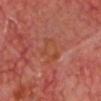Q: How was the tile lit?
A: cross-polarized illumination
Q: What are the patient's age and sex?
A: male, in their mid-60s
Q: What is the lesion's diameter?
A: ≈4 mm
Q: What kind of image is this?
A: 15 mm crop, total-body photography
Q: What is the anatomic site?
A: the head or neck